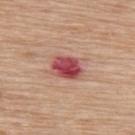biopsy status: no biopsy performed (imaged during a skin exam)
body site: the back
lighting: white-light illumination
lesion diameter: ≈3.5 mm
automated lesion analysis: a lesion area of about 8 mm², an outline eccentricity of about 0.7 (0 = round, 1 = elongated), and a shape-asymmetry score of about 0.15 (0 = symmetric); about 16 CIELAB-L* units darker than the surrounding skin and a lesion-to-skin contrast of about 11.5 (normalized; higher = more distinct)
subject: female, in their mid- to late 60s
acquisition: ~15 mm tile from a whole-body skin photo